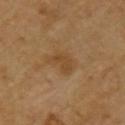Findings:
• notes: catalogued during a skin exam; not biopsied
• illumination: cross-polarized
• acquisition: 15 mm crop, total-body photography
• diameter: ≈3.5 mm
• site: the chest
• subject: male, roughly 65 years of age
• image-analysis metrics: a border-irregularity rating of about 4/10; an automated nevus-likeness rating near 5 out of 100 and a detector confidence of about 100 out of 100 that the crop contains a lesion